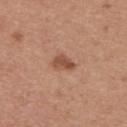  biopsy_status: not biopsied; imaged during a skin examination
  image:
    source: total-body photography crop
    field_of_view_mm: 15
  site: upper back
  patient:
    sex: female
    age_approx: 35